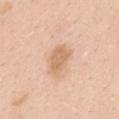Q: Was this lesion biopsied?
A: imaged on a skin check; not biopsied
Q: What are the patient's age and sex?
A: female, aged 38 to 42
Q: How was this image acquired?
A: ~15 mm crop, total-body skin-cancer survey
Q: What is the anatomic site?
A: the mid back